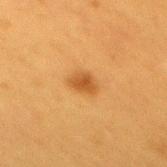<tbp_lesion>
  <lighting>cross-polarized</lighting>
  <image>
    <source>total-body photography crop</source>
    <field_of_view_mm>15</field_of_view_mm>
  </image>
  <site>mid back</site>
  <patient>
    <sex>female</sex>
    <age_approx>40</age_approx>
  </patient>
</tbp_lesion>